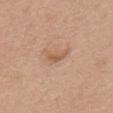The lesion was photographed on a routine skin check and not biopsied; there is no pathology result.
Automated tile analysis of the lesion measured internal color variation of about 2 on a 0–10 scale and a peripheral color-asymmetry measure near 1.
Imaged with white-light lighting.
A region of skin cropped from a whole-body photographic capture, roughly 15 mm wide.
From the chest.
The recorded lesion diameter is about 3 mm.
A female patient, approximately 60 years of age.